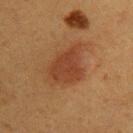Part of a total-body skin-imaging series; this lesion was reviewed on a skin check and was not flagged for biopsy.
A female subject in their 40s.
About 5.5 mm across.
Automated tile analysis of the lesion measured an area of roughly 14 mm², an outline eccentricity of about 0.7 (0 = round, 1 = elongated), and two-axis asymmetry of about 0.25. And it measured roughly 7 lightness units darker than nearby skin and a normalized border contrast of about 7. The analysis additionally found an automated nevus-likeness rating near 95 out of 100 and lesion-presence confidence of about 100/100.
The tile uses cross-polarized illumination.
The lesion is located on the arm.
This image is a 15 mm lesion crop taken from a total-body photograph.